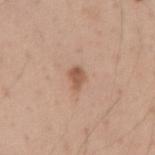The lesion was tiled from a total-body skin photograph and was not biopsied. The subject is a male roughly 40 years of age. The lesion is on the right upper arm. An algorithmic analysis of the crop reported border irregularity of about 2.5 on a 0–10 scale and radial color variation of about 0.5. The software also gave an automated nevus-likeness rating near 80 out of 100 and a lesion-detection confidence of about 100/100. A lesion tile, about 15 mm wide, cut from a 3D total-body photograph. This is a white-light tile. The recorded lesion diameter is about 2.5 mm.